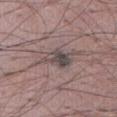notes = imaged on a skin check; not biopsied | location = the abdomen | lighting = white-light | subject = male, approximately 70 years of age | image = total-body-photography crop, ~15 mm field of view.